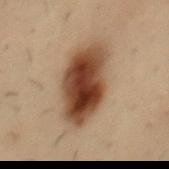{
  "site": "mid back",
  "image": {
    "source": "total-body photography crop",
    "field_of_view_mm": 15
  },
  "patient": {
    "sex": "male",
    "age_approx": 30
  },
  "lesion_size": {
    "long_diameter_mm_approx": 7.5
  },
  "lighting": "cross-polarized"
}